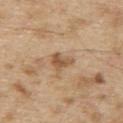workup: no biopsy performed (imaged during a skin exam); location: the upper back; imaging modality: ~15 mm crop, total-body skin-cancer survey; patient: male, aged 68–72.